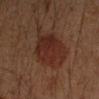Clinical summary:
A 15 mm crop from a total-body photograph taken for skin-cancer surveillance. This is a cross-polarized tile. The total-body-photography lesion software estimated a classifier nevus-likeness of about 100/100 and a detector confidence of about 100 out of 100 that the crop contains a lesion. Longest diameter approximately 6 mm. On the left forearm. The patient is a male approximately 50 years of age.